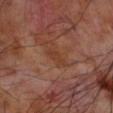<case>
  <biopsy_status>not biopsied; imaged during a skin examination</biopsy_status>
  <image>
    <source>total-body photography crop</source>
    <field_of_view_mm>15</field_of_view_mm>
  </image>
  <lighting>cross-polarized</lighting>
  <site>left forearm</site>
  <patient>
    <sex>male</sex>
    <age_approx>70</age_approx>
  </patient>
  <lesion_size>
    <long_diameter_mm_approx>3.0</long_diameter_mm_approx>
  </lesion_size>
</case>